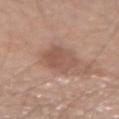Imaged during a routine full-body skin examination; the lesion was not biopsied and no histopathology is available. About 3.5 mm across. Automated tile analysis of the lesion measured a lesion area of about 9 mm², a shape eccentricity near 0.5, and a symmetry-axis asymmetry near 0.15. It also reported an average lesion color of about L≈53 a*≈20 b*≈27 (CIELAB), a lesion–skin lightness drop of about 8, and a normalized border contrast of about 6. The analysis additionally found border irregularity of about 2 on a 0–10 scale, internal color variation of about 3 on a 0–10 scale, and a peripheral color-asymmetry measure near 1. Cropped from a whole-body photographic skin survey; the tile spans about 15 mm. From the left forearm. Imaged with white-light lighting. A male subject aged 58 to 62.